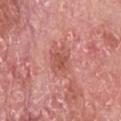The lesion was photographed on a routine skin check and not biopsied; there is no pathology result.